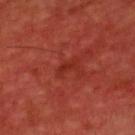Findings:
- workup · total-body-photography surveillance lesion; no biopsy
- TBP lesion metrics · a peripheral color-asymmetry measure near 0
- image · ~15 mm crop, total-body skin-cancer survey
- subject · male, aged around 60
- tile lighting · cross-polarized illumination
- diameter · about 3 mm
- location · the front of the torso